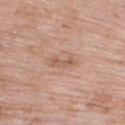Cropped from a whole-body photographic skin survey; the tile spans about 15 mm.
The lesion is on the right upper arm.
Automated tile analysis of the lesion measured an area of roughly 2.5 mm², an outline eccentricity of about 0.95 (0 = round, 1 = elongated), and a symmetry-axis asymmetry near 0.35. And it measured border irregularity of about 4.5 on a 0–10 scale and a within-lesion color-variation index near 0/10.
The tile uses white-light illumination.
The patient is a female about 70 years old.